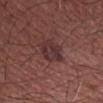biopsy_status: not biopsied; imaged during a skin examination
site: arm
lighting: white-light
lesion_size:
  long_diameter_mm_approx: 3.5
patient:
  sex: male
  age_approx: 70
image:
  source: total-body photography crop
  field_of_view_mm: 15